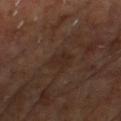Part of a total-body skin-imaging series; this lesion was reviewed on a skin check and was not flagged for biopsy. Captured under cross-polarized illumination. The patient is a male approximately 60 years of age. The lesion is on the back. Cropped from a whole-body photographic skin survey; the tile spans about 15 mm.